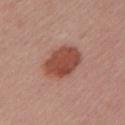No biopsy was performed on this lesion — it was imaged during a full skin examination and was not determined to be concerning.
Automated tile analysis of the lesion measured a footprint of about 14 mm² and a shape-asymmetry score of about 0.15 (0 = symmetric). The software also gave a lesion color around L≈48 a*≈26 b*≈28 in CIELAB, about 13 CIELAB-L* units darker than the surrounding skin, and a normalized border contrast of about 9.5. And it measured border irregularity of about 1.5 on a 0–10 scale. It also reported a classifier nevus-likeness of about 100/100 and a detector confidence of about 100 out of 100 that the crop contains a lesion.
Cropped from a whole-body photographic skin survey; the tile spans about 15 mm.
The patient is a female roughly 55 years of age.
On the left upper arm.